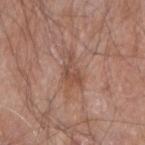{
  "biopsy_status": "not biopsied; imaged during a skin examination"
}